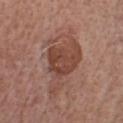Recorded during total-body skin imaging; not selected for excision or biopsy. Cropped from a total-body skin-imaging series; the visible field is about 15 mm. On the chest. A male subject, approximately 55 years of age. Captured under white-light illumination.